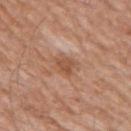The lesion is on the left upper arm.
A roughly 15 mm field-of-view crop from a total-body skin photograph.
A male patient aged approximately 60.